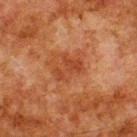Assessment:
Recorded during total-body skin imaging; not selected for excision or biopsy.
Context:
The patient is a male aged 78 to 82. Measured at roughly 3.5 mm in maximum diameter. A 15 mm close-up extracted from a 3D total-body photography capture. On the upper back. The total-body-photography lesion software estimated an average lesion color of about L≈35 a*≈24 b*≈31 (CIELAB), about 6 CIELAB-L* units darker than the surrounding skin, and a normalized lesion–skin contrast near 6. And it measured an automated nevus-likeness rating near 0 out of 100 and lesion-presence confidence of about 100/100.